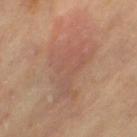Q: Was a biopsy performed?
A: no biopsy performed (imaged during a skin exam)
Q: Automated lesion metrics?
A: a lesion area of about 23 mm², an eccentricity of roughly 0.8, and two-axis asymmetry of about 0.5; a lesion color around L≈55 a*≈21 b*≈28 in CIELAB, about 6 CIELAB-L* units darker than the surrounding skin, and a normalized lesion–skin contrast near 4.5; a nevus-likeness score of about 0/100 and a detector confidence of about 95 out of 100 that the crop contains a lesion
Q: Where on the body is the lesion?
A: the left thigh
Q: What is the lesion's diameter?
A: ~7.5 mm (longest diameter)
Q: What are the patient's age and sex?
A: female, aged 68 to 72
Q: How was the tile lit?
A: cross-polarized illumination
Q: What kind of image is this?
A: total-body-photography crop, ~15 mm field of view